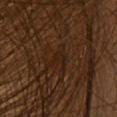| field | value |
|---|---|
| illumination | cross-polarized illumination |
| anatomic site | the head or neck |
| automated metrics | a lesion area of about 4.5 mm² and a shape-asymmetry score of about 0.65 (0 = symmetric); an average lesion color of about L≈21 a*≈16 b*≈24 (CIELAB) and a normalized border contrast of about 5.5; a border-irregularity index near 7.5/10 and a within-lesion color-variation index near 1.5/10; an automated nevus-likeness rating near 0 out of 100 and a lesion-detection confidence of about 0/100 |
| image | ~15 mm crop, total-body skin-cancer survey |
| lesion diameter | ~4 mm (longest diameter) |
| subject | female, aged 28–32 |